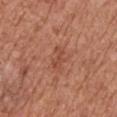| field | value |
|---|---|
| notes | total-body-photography surveillance lesion; no biopsy |
| lighting | white-light illumination |
| lesion size | ~3 mm (longest diameter) |
| anatomic site | the right upper arm |
| subject | male, in their mid- to late 70s |
| image | total-body-photography crop, ~15 mm field of view |